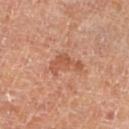Q: Is there a histopathology result?
A: no biopsy performed (imaged during a skin exam)